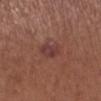Clinical impression:
Captured during whole-body skin photography for melanoma surveillance; the lesion was not biopsied.
Acquisition and patient details:
A female subject aged approximately 55. A 15 mm close-up tile from a total-body photography series done for melanoma screening. From the left lower leg.